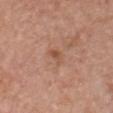Recorded during total-body skin imaging; not selected for excision or biopsy.
Automated image analysis of the tile measured a shape eccentricity near 0.75 and two-axis asymmetry of about 0.25.
Approximately 3 mm at its widest.
This image is a 15 mm lesion crop taken from a total-body photograph.
The subject is a male approximately 60 years of age.
Located on the chest.
Imaged with white-light lighting.